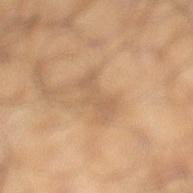The lesion was tiled from a total-body skin photograph and was not biopsied. This is a cross-polarized tile. Approximately 4.5 mm at its widest. A male patient aged 63–67. A lesion tile, about 15 mm wide, cut from a 3D total-body photograph. From the left lower leg.